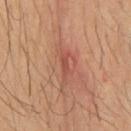{"biopsy_status": "not biopsied; imaged during a skin examination", "site": "chest", "patient": {"sex": "male", "age_approx": 60}, "image": {"source": "total-body photography crop", "field_of_view_mm": 15}}